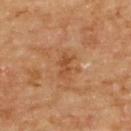The lesion was photographed on a routine skin check and not biopsied; there is no pathology result. A male subject, aged around 85 or older. About 2.5 mm across. A close-up tile cropped from a whole-body skin photograph, about 15 mm across. From the upper back. The total-body-photography lesion software estimated a lesion area of about 3 mm², a shape eccentricity near 0.8, and two-axis asymmetry of about 0.35. It also reported about 8 CIELAB-L* units darker than the surrounding skin. Imaged with cross-polarized lighting.